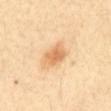* workup — catalogued during a skin exam; not biopsied
* location — the abdomen
* subject — female, roughly 35 years of age
* diameter — ≈3 mm
* image source — total-body-photography crop, ~15 mm field of view
* tile lighting — cross-polarized